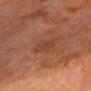Part of a total-body skin-imaging series; this lesion was reviewed on a skin check and was not flagged for biopsy. A 15 mm close-up extracted from a 3D total-body photography capture. A male patient roughly 75 years of age. The lesion is on the chest.